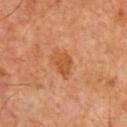Q: Was a biopsy performed?
A: no biopsy performed (imaged during a skin exam)
Q: How was this image acquired?
A: ~15 mm crop, total-body skin-cancer survey
Q: What is the anatomic site?
A: the chest
Q: Patient demographics?
A: male, in their mid- to late 60s